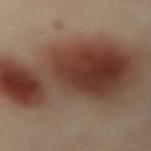Part of a total-body skin-imaging series; this lesion was reviewed on a skin check and was not flagged for biopsy. A female subject, about 55 years old. The lesion is on the lower back. Automated image analysis of the tile measured a classifier nevus-likeness of about 100/100. The recorded lesion diameter is about 13.5 mm. A 15 mm close-up tile from a total-body photography series done for melanoma screening.